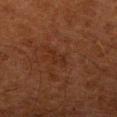Case summary:
* follow-up — total-body-photography surveillance lesion; no biopsy
* patient — male, aged 78 to 82
* image — ~15 mm crop, total-body skin-cancer survey
* anatomic site — the right lower leg
* lesion size — ≈3 mm